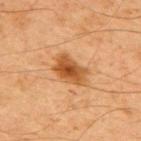{
  "biopsy_status": "not biopsied; imaged during a skin examination",
  "site": "upper back",
  "lighting": "cross-polarized",
  "image": {
    "source": "total-body photography crop",
    "field_of_view_mm": 15
  },
  "patient": {
    "sex": "male",
    "age_approx": 65
  }
}